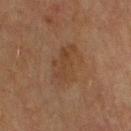Q: Was this lesion biopsied?
A: imaged on a skin check; not biopsied
Q: Lesion location?
A: the right upper arm
Q: Automated lesion metrics?
A: a mean CIELAB color near L≈34 a*≈15 b*≈26, roughly 5 lightness units darker than nearby skin, and a lesion-to-skin contrast of about 5 (normalized; higher = more distinct); a border-irregularity index near 4/10, internal color variation of about 2.5 on a 0–10 scale, and a peripheral color-asymmetry measure near 1; a classifier nevus-likeness of about 0/100 and a detector confidence of about 100 out of 100 that the crop contains a lesion
Q: Illumination type?
A: cross-polarized
Q: What is the imaging modality?
A: 15 mm crop, total-body photography
Q: Patient demographics?
A: male, roughly 75 years of age